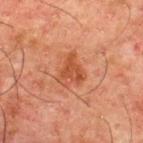| key | value |
|---|---|
| TBP lesion metrics | an area of roughly 7.5 mm², an eccentricity of roughly 0.6, and a shape-asymmetry score of about 0.5 (0 = symmetric); a border-irregularity rating of about 5/10 and internal color variation of about 3 on a 0–10 scale; a nevus-likeness score of about 25/100 and lesion-presence confidence of about 100/100 |
| patient | male, aged 48–52 |
| tile lighting | cross-polarized illumination |
| lesion diameter | about 4 mm |
| image source | total-body-photography crop, ~15 mm field of view |
| body site | the upper back |